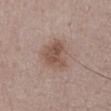Assessment: Imaged during a routine full-body skin examination; the lesion was not biopsied and no histopathology is available. Image and clinical context: Approximately 4.5 mm at its widest. Captured under white-light illumination. This image is a 15 mm lesion crop taken from a total-body photograph. Located on the abdomen. A male patient, in their mid-50s.